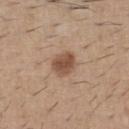Captured during whole-body skin photography for melanoma surveillance; the lesion was not biopsied. Located on the chest. Longest diameter approximately 3 mm. Cropped from a total-body skin-imaging series; the visible field is about 15 mm. A male patient, about 60 years old.